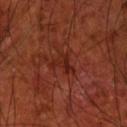This lesion was catalogued during total-body skin photography and was not selected for biopsy.
A male subject aged 68–72.
A 15 mm close-up extracted from a 3D total-body photography capture.
The lesion's longest dimension is about 3.5 mm.
Located on the front of the torso.
Captured under cross-polarized illumination.